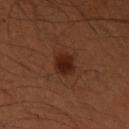notes: catalogued during a skin exam; not biopsied | location: the arm | image source: total-body-photography crop, ~15 mm field of view | lesion diameter: ~3 mm (longest diameter) | patient: male, in their mid-30s | illumination: cross-polarized | image-analysis metrics: an area of roughly 5.5 mm² and two-axis asymmetry of about 0.2; a border-irregularity rating of about 2/10, a within-lesion color-variation index near 3/10, and peripheral color asymmetry of about 1; a nevus-likeness score of about 95/100 and lesion-presence confidence of about 100/100.